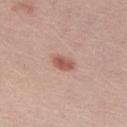{
  "biopsy_status": "not biopsied; imaged during a skin examination",
  "patient": {
    "sex": "male",
    "age_approx": 30
  },
  "lighting": "white-light",
  "lesion_size": {
    "long_diameter_mm_approx": 2.5
  },
  "automated_metrics": {
    "cielab_L": 55,
    "cielab_a": 25,
    "cielab_b": 26,
    "vs_skin_darker_L": 12.0,
    "vs_skin_contrast_norm": 8.0,
    "border_irregularity_0_10": 2.5,
    "color_variation_0_10": 2.5,
    "peripheral_color_asymmetry": 0.5,
    "nevus_likeness_0_100": 85,
    "lesion_detection_confidence_0_100": 100
  },
  "image": {
    "source": "total-body photography crop",
    "field_of_view_mm": 15
  }
}